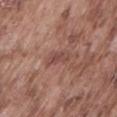Clinical impression: Imaged during a routine full-body skin examination; the lesion was not biopsied and no histopathology is available. Context: The lesion's longest dimension is about 2.5 mm. The total-body-photography lesion software estimated a footprint of about 3 mm² and a shape eccentricity near 0.85. It also reported a lesion color around L≈46 a*≈23 b*≈24 in CIELAB, a lesion–skin lightness drop of about 8, and a normalized lesion–skin contrast near 6. The software also gave a border-irregularity rating of about 4.5/10, a color-variation rating of about 0/10, and radial color variation of about 0. The lesion is on the lower back. A lesion tile, about 15 mm wide, cut from a 3D total-body photograph. A male subject aged around 75. This is a white-light tile.